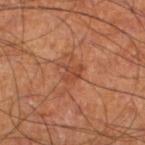Q: Is there a histopathology result?
A: imaged on a skin check; not biopsied
Q: Where on the body is the lesion?
A: the leg
Q: Who is the patient?
A: male, aged approximately 60
Q: What did automated image analysis measure?
A: a lesion area of about 3.5 mm²; a border-irregularity rating of about 5/10, a within-lesion color-variation index near 2.5/10, and a peripheral color-asymmetry measure near 1
Q: Lesion size?
A: about 2.5 mm
Q: What is the imaging modality?
A: total-body-photography crop, ~15 mm field of view
Q: How was the tile lit?
A: cross-polarized